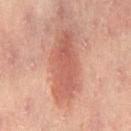follow-up: total-body-photography surveillance lesion; no biopsy.